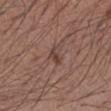{
  "biopsy_status": "not biopsied; imaged during a skin examination",
  "image": {
    "source": "total-body photography crop",
    "field_of_view_mm": 15
  },
  "site": "left forearm",
  "lighting": "white-light",
  "patient": {
    "sex": "male",
    "age_approx": 35
  },
  "lesion_size": {
    "long_diameter_mm_approx": 2.5
  }
}